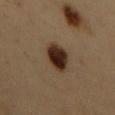{"biopsy_status": "not biopsied; imaged during a skin examination", "lighting": "cross-polarized", "automated_metrics": {"area_mm2_approx": 9.5, "eccentricity": 0.8, "shape_asymmetry": 0.1, "cielab_L": 29, "cielab_a": 16, "cielab_b": 25, "vs_skin_darker_L": 16.0, "vs_skin_contrast_norm": 14.5, "lesion_detection_confidence_0_100": 100}, "image": {"source": "total-body photography crop", "field_of_view_mm": 15}, "site": "back", "patient": {"sex": "male", "age_approx": 50}, "lesion_size": {"long_diameter_mm_approx": 4.5}}